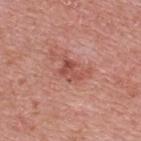Recorded during total-body skin imaging; not selected for excision or biopsy.
The tile uses white-light illumination.
Located on the upper back.
A region of skin cropped from a whole-body photographic capture, roughly 15 mm wide.
The lesion-visualizer software estimated a lesion area of about 5 mm², an outline eccentricity of about 0.7 (0 = round, 1 = elongated), and a shape-asymmetry score of about 0.4 (0 = symmetric). The analysis additionally found about 9 CIELAB-L* units darker than the surrounding skin and a lesion-to-skin contrast of about 6.5 (normalized; higher = more distinct). The analysis additionally found a detector confidence of about 100 out of 100 that the crop contains a lesion.
The subject is a male in their 70s.
The lesion's longest dimension is about 3 mm.